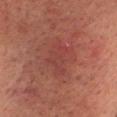* location — the head or neck
* illumination — cross-polarized illumination
* patient — male, aged 48 to 52
* image — ~15 mm tile from a whole-body skin photo
* size — ≈5 mm